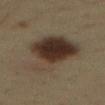Impression: Part of a total-body skin-imaging series; this lesion was reviewed on a skin check and was not flagged for biopsy. Acquisition and patient details: An algorithmic analysis of the crop reported a footprint of about 32 mm², an outline eccentricity of about 0.85 (0 = round, 1 = elongated), and two-axis asymmetry of about 0.35. And it measured border irregularity of about 4.5 on a 0–10 scale, internal color variation of about 8.5 on a 0–10 scale, and peripheral color asymmetry of about 2.5. A male patient, aged 33 to 37. The lesion is located on the abdomen. The tile uses cross-polarized illumination. Longest diameter approximately 9.5 mm. This image is a 15 mm lesion crop taken from a total-body photograph.